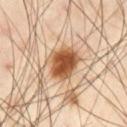<record>
<biopsy_status>not biopsied; imaged during a skin examination</biopsy_status>
<lighting>cross-polarized</lighting>
<automated_metrics>
  <area_mm2_approx>13.0</area_mm2_approx>
  <vs_skin_darker_L>18.0</vs_skin_darker_L>
  <border_irregularity_0_10>2.5</border_irregularity_0_10>
  <color_variation_0_10>6.5</color_variation_0_10>
  <nevus_likeness_0_100>100</nevus_likeness_0_100>
  <lesion_detection_confidence_0_100>100</lesion_detection_confidence_0_100>
</automated_metrics>
<image>
  <source>total-body photography crop</source>
  <field_of_view_mm>15</field_of_view_mm>
</image>
<lesion_size>
  <long_diameter_mm_approx>4.5</long_diameter_mm_approx>
</lesion_size>
<site>left thigh</site>
<patient>
  <sex>male</sex>
  <age_approx>55</age_approx>
</patient>
</record>